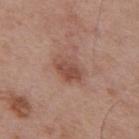Acquisition and patient details: The total-body-photography lesion software estimated two-axis asymmetry of about 0.25. The software also gave an average lesion color of about L≈50 a*≈21 b*≈27 (CIELAB) and roughly 9 lightness units darker than nearby skin. The analysis additionally found border irregularity of about 2.5 on a 0–10 scale, a color-variation rating of about 4/10, and peripheral color asymmetry of about 1.5. The lesion is on the mid back. Cropped from a total-body skin-imaging series; the visible field is about 15 mm. Measured at roughly 4 mm in maximum diameter. Imaged with white-light lighting. A male subject aged 53 to 57.